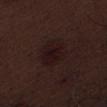Case summary:
– follow-up: no biopsy performed (imaged during a skin exam)
– automated lesion analysis: a color-variation rating of about 3/10 and radial color variation of about 1; a classifier nevus-likeness of about 0/100 and lesion-presence confidence of about 100/100
– lesion diameter: about 4.5 mm
– image source: total-body-photography crop, ~15 mm field of view
– site: the right thigh
– subject: male, roughly 70 years of age